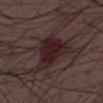Findings:
– workup — imaged on a skin check; not biopsied
– patient — male, aged 48 to 52
– lesion size — about 4.5 mm
– imaging modality — ~15 mm crop, total-body skin-cancer survey
– automated lesion analysis — a border-irregularity index near 4/10 and a color-variation rating of about 4.5/10; a detector confidence of about 100 out of 100 that the crop contains a lesion